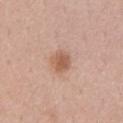Impression:
The lesion was photographed on a routine skin check and not biopsied; there is no pathology result.
Image and clinical context:
Automated image analysis of the tile measured a lesion color around L≈58 a*≈21 b*≈30 in CIELAB, roughly 10 lightness units darker than nearby skin, and a normalized border contrast of about 7.5. The software also gave internal color variation of about 2.5 on a 0–10 scale and peripheral color asymmetry of about 1. A male patient about 55 years old. About 3 mm across. A roughly 15 mm field-of-view crop from a total-body skin photograph.